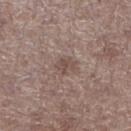biopsy_status: not biopsied; imaged during a skin examination
lesion_size:
  long_diameter_mm_approx: 2.5
automated_metrics:
  area_mm2_approx: 4.0
  eccentricity: 0.55
  shape_asymmetry: 0.3
  border_irregularity_0_10: 3.0
  color_variation_0_10: 1.5
  peripheral_color_asymmetry: 0.5
  nevus_likeness_0_100: 0
  lesion_detection_confidence_0_100: 100
site: right lower leg
image:
  source: total-body photography crop
  field_of_view_mm: 15
patient:
  sex: male
  age_approx: 70